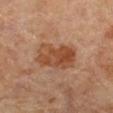Part of a total-body skin-imaging series; this lesion was reviewed on a skin check and was not flagged for biopsy. Approximately 5.5 mm at its widest. This image is a 15 mm lesion crop taken from a total-body photograph. From the right lower leg. Imaged with cross-polarized lighting. An algorithmic analysis of the crop reported an average lesion color of about L≈45 a*≈22 b*≈32 (CIELAB), roughly 10 lightness units darker than nearby skin, and a lesion-to-skin contrast of about 8 (normalized; higher = more distinct). The analysis additionally found internal color variation of about 5.5 on a 0–10 scale and a peripheral color-asymmetry measure near 2. A female patient in their 70s.